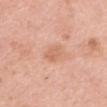Notes:
* follow-up · no biopsy performed (imaged during a skin exam)
* lesion size · ~3 mm (longest diameter)
* image source · ~15 mm tile from a whole-body skin photo
* subject · female, aged 38–42
* lighting · white-light
* anatomic site · the upper back
* image-analysis metrics · a lesion color around L≈64 a*≈24 b*≈33 in CIELAB, roughly 7 lightness units darker than nearby skin, and a normalized border contrast of about 5.5; a border-irregularity rating of about 2.5/10; a classifier nevus-likeness of about 0/100 and a lesion-detection confidence of about 100/100